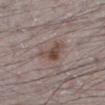follow-up — imaged on a skin check; not biopsied | image-analysis metrics — a lesion color around L≈47 a*≈16 b*≈21 in CIELAB, roughly 9 lightness units darker than nearby skin, and a normalized border contrast of about 8; a nevus-likeness score of about 85/100 and a detector confidence of about 100 out of 100 that the crop contains a lesion | lighting — white-light | imaging modality — 15 mm crop, total-body photography | site — the left lower leg | patient — male, aged 48 to 52.